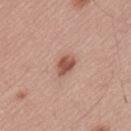Impression:
No biopsy was performed on this lesion — it was imaged during a full skin examination and was not determined to be concerning.
Background:
Located on the back. A male patient in their mid- to late 50s. A 15 mm close-up extracted from a 3D total-body photography capture.